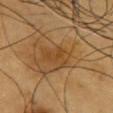This lesion was catalogued during total-body skin photography and was not selected for biopsy. Cropped from a whole-body photographic skin survey; the tile spans about 15 mm. The lesion's longest dimension is about 3.5 mm. The lesion is on the chest. A male patient, approximately 60 years of age.